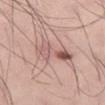The lesion was tiled from a total-body skin photograph and was not biopsied. Longest diameter approximately 5.5 mm. Cropped from a whole-body photographic skin survey; the tile spans about 15 mm. This is a white-light tile. A male patient, aged 33–37. On the left thigh.